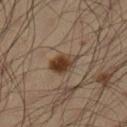The lesion was photographed on a routine skin check and not biopsied; there is no pathology result. Automated tile analysis of the lesion measured an area of roughly 6.5 mm², a shape eccentricity near 0.8, and two-axis asymmetry of about 0.25. And it measured an average lesion color of about L≈34 a*≈15 b*≈26 (CIELAB), a lesion–skin lightness drop of about 12, and a normalized lesion–skin contrast near 11. The analysis additionally found an automated nevus-likeness rating near 95 out of 100 and a lesion-detection confidence of about 100/100. The patient is a male in their mid-30s. This image is a 15 mm lesion crop taken from a total-body photograph. The tile uses cross-polarized illumination. Measured at roughly 4 mm in maximum diameter. On the left lower leg.